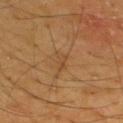workup: total-body-photography surveillance lesion; no biopsy | image: ~15 mm tile from a whole-body skin photo | body site: the upper back | tile lighting: cross-polarized | lesion diameter: ~2.5 mm (longest diameter) | patient: male, roughly 65 years of age.